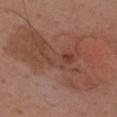Imaged during a routine full-body skin examination; the lesion was not biopsied and no histopathology is available.
A lesion tile, about 15 mm wide, cut from a 3D total-body photograph.
Captured under white-light illumination.
The lesion is on the upper back.
The subject is a male about 40 years old.
About 12.5 mm across.
The total-body-photography lesion software estimated about 7 CIELAB-L* units darker than the surrounding skin. The analysis additionally found a border-irregularity index near 8.5/10 and a peripheral color-asymmetry measure near 1.5. The analysis additionally found lesion-presence confidence of about 100/100.